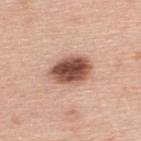Assessment:
The lesion was photographed on a routine skin check and not biopsied; there is no pathology result.
Background:
Located on the upper back. A 15 mm close-up extracted from a 3D total-body photography capture. The subject is a male about 50 years old.